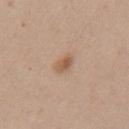Clinical impression:
Part of a total-body skin-imaging series; this lesion was reviewed on a skin check and was not flagged for biopsy.
Context:
A female subject, aged 38–42. The lesion is located on the back. A close-up tile cropped from a whole-body skin photograph, about 15 mm across. Imaged with white-light lighting. Measured at roughly 3 mm in maximum diameter.